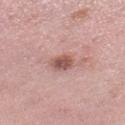Part of a total-body skin-imaging series; this lesion was reviewed on a skin check and was not flagged for biopsy.
Longest diameter approximately 2.5 mm.
A lesion tile, about 15 mm wide, cut from a 3D total-body photograph.
The lesion-visualizer software estimated a lesion-to-skin contrast of about 8.5 (normalized; higher = more distinct). The analysis additionally found a peripheral color-asymmetry measure near 1.5. The software also gave an automated nevus-likeness rating near 80 out of 100.
From the left lower leg.
A female subject aged 48–52.